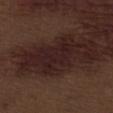workup = no biopsy performed (imaged during a skin exam) | imaging modality = ~15 mm tile from a whole-body skin photo | size = ≈10.5 mm | patient = male, roughly 70 years of age | tile lighting = white-light | automated metrics = a classifier nevus-likeness of about 0/100 and a lesion-detection confidence of about 70/100 | body site = the abdomen.